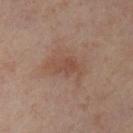No biopsy was performed on this lesion — it was imaged during a full skin examination and was not determined to be concerning.
The lesion is located on the leg.
Longest diameter approximately 4.5 mm.
A female patient, approximately 55 years of age.
A close-up tile cropped from a whole-body skin photograph, about 15 mm across.
Imaged with cross-polarized lighting.